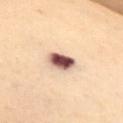Assessment: Imaged during a routine full-body skin examination; the lesion was not biopsied and no histopathology is available. Acquisition and patient details: Captured under cross-polarized illumination. From the chest. A female subject roughly 50 years of age. This image is a 15 mm lesion crop taken from a total-body photograph.